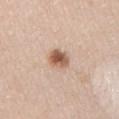{"biopsy_status": "not biopsied; imaged during a skin examination", "site": "left upper arm", "image": {"source": "total-body photography crop", "field_of_view_mm": 15}, "lighting": "white-light", "automated_metrics": {"cielab_L": 58, "cielab_a": 19, "cielab_b": 31, "vs_skin_darker_L": 15.0, "vs_skin_contrast_norm": 9.5, "border_irregularity_0_10": 1.5, "color_variation_0_10": 5.5, "peripheral_color_asymmetry": 2.0, "nevus_likeness_0_100": 100}, "patient": {"sex": "female", "age_approx": 55}}